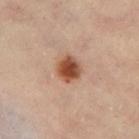No biopsy was performed on this lesion — it was imaged during a full skin examination and was not determined to be concerning. The total-body-photography lesion software estimated border irregularity of about 2 on a 0–10 scale. The recorded lesion diameter is about 3 mm. A 15 mm close-up extracted from a 3D total-body photography capture. The subject is a female aged 58 to 62. From the left thigh. This is a cross-polarized tile.